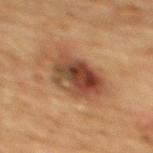This lesion was catalogued during total-body skin photography and was not selected for biopsy. The tile uses cross-polarized illumination. An algorithmic analysis of the crop reported an outline eccentricity of about 0.7 (0 = round, 1 = elongated). The analysis additionally found border irregularity of about 2.5 on a 0–10 scale, a color-variation rating of about 9/10, and a peripheral color-asymmetry measure near 3. And it measured a classifier nevus-likeness of about 30/100 and lesion-presence confidence of about 100/100. Cropped from a whole-body photographic skin survey; the tile spans about 15 mm. The lesion's longest dimension is about 6.5 mm. The lesion is on the upper back. A female subject aged around 70.